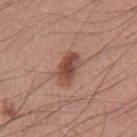Impression:
No biopsy was performed on this lesion — it was imaged during a full skin examination and was not determined to be concerning.
Acquisition and patient details:
The tile uses white-light illumination. Cropped from a whole-body photographic skin survey; the tile spans about 15 mm. The lesion's longest dimension is about 4 mm. Automated image analysis of the tile measured a footprint of about 8 mm² and an eccentricity of roughly 0.8. The analysis additionally found a lesion color around L≈48 a*≈22 b*≈27 in CIELAB, a lesion–skin lightness drop of about 12, and a normalized border contrast of about 8.5. The software also gave border irregularity of about 2.5 on a 0–10 scale. The analysis additionally found an automated nevus-likeness rating near 80 out of 100 and a detector confidence of about 100 out of 100 that the crop contains a lesion. On the right upper arm. A male subject, roughly 40 years of age.